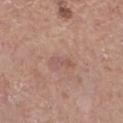Clinical impression: The lesion was tiled from a total-body skin photograph and was not biopsied. Context: The patient is a male aged approximately 50. The lesion is located on the left lower leg. A 15 mm close-up extracted from a 3D total-body photography capture.